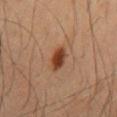biopsy status — imaged on a skin check; not biopsied
illumination — cross-polarized
subject — male, in their mid- to late 40s
acquisition — ~15 mm tile from a whole-body skin photo
location — the mid back
size — ≈3.5 mm